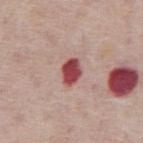Impression:
Recorded during total-body skin imaging; not selected for excision or biopsy.
Acquisition and patient details:
A male subject, aged 73–77. On the abdomen. Captured under white-light illumination. About 3 mm across. A 15 mm crop from a total-body photograph taken for skin-cancer surveillance. An algorithmic analysis of the crop reported a lesion area of about 5.5 mm², a shape eccentricity near 0.7, and a shape-asymmetry score of about 0.15 (0 = symmetric). The software also gave a mean CIELAB color near L≈47 a*≈33 b*≈21 and a lesion–skin lightness drop of about 19. The analysis additionally found a nevus-likeness score of about 0/100 and a detector confidence of about 100 out of 100 that the crop contains a lesion.